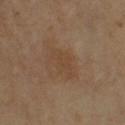  biopsy_status: not biopsied; imaged during a skin examination
  lighting: cross-polarized
  image:
    source: total-body photography crop
    field_of_view_mm: 15
  lesion_size:
    long_diameter_mm_approx: 4.5
  patient:
    sex: male
    age_approx: 70
  site: right upper arm
  automated_metrics:
    area_mm2_approx: 7.0
    eccentricity: 0.85
    cielab_L: 41
    cielab_a: 16
    cielab_b: 29
    vs_skin_contrast_norm: 4.5
    border_irregularity_0_10: 4.5
    color_variation_0_10: 1.0